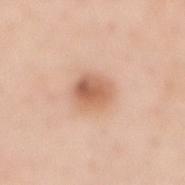The lesion was photographed on a routine skin check and not biopsied; there is no pathology result.
On the mid back.
The subject is a female approximately 50 years of age.
The recorded lesion diameter is about 3.5 mm.
The tile uses white-light illumination.
A 15 mm close-up tile from a total-body photography series done for melanoma screening.